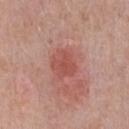Captured during whole-body skin photography for melanoma surveillance; the lesion was not biopsied. Captured under white-light illumination. A 15 mm crop from a total-body photograph taken for skin-cancer surveillance. Measured at roughly 3.5 mm in maximum diameter. The total-body-photography lesion software estimated a lesion area of about 9 mm² and a shape eccentricity near 0.45. And it measured a lesion color around L≈52 a*≈28 b*≈27 in CIELAB and a lesion–skin lightness drop of about 8. The analysis additionally found border irregularity of about 1.5 on a 0–10 scale, a color-variation rating of about 2.5/10, and radial color variation of about 1. The lesion is on the chest. The subject is a male aged 48–52.